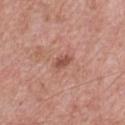Imaged during a routine full-body skin examination; the lesion was not biopsied and no histopathology is available.
Approximately 2.5 mm at its widest.
The total-body-photography lesion software estimated a footprint of about 3 mm², an eccentricity of roughly 0.8, and a symmetry-axis asymmetry near 0.25. The software also gave a lesion–skin lightness drop of about 10 and a lesion-to-skin contrast of about 7 (normalized; higher = more distinct).
The patient is a male roughly 50 years of age.
From the chest.
Cropped from a total-body skin-imaging series; the visible field is about 15 mm.
This is a white-light tile.